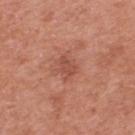{"biopsy_status": "not biopsied; imaged during a skin examination"}